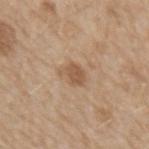Q: Was a biopsy performed?
A: total-body-photography surveillance lesion; no biopsy